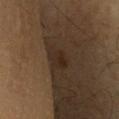Part of a total-body skin-imaging series; this lesion was reviewed on a skin check and was not flagged for biopsy. The lesion is located on the chest. A close-up tile cropped from a whole-body skin photograph, about 15 mm across. The patient is a male in their 60s.